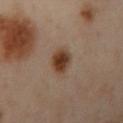Clinical impression: No biopsy was performed on this lesion — it was imaged during a full skin examination and was not determined to be concerning. Background: Cropped from a total-body skin-imaging series; the visible field is about 15 mm. The subject is a female aged 38 to 42. The recorded lesion diameter is about 3 mm. On the left arm.